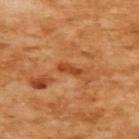The lesion was photographed on a routine skin check and not biopsied; there is no pathology result. Cropped from a whole-body photographic skin survey; the tile spans about 15 mm. A female patient approximately 55 years of age. The lesion is on the back. Automated image analysis of the tile measured a border-irregularity rating of about 3.5/10, internal color variation of about 0 on a 0–10 scale, and radial color variation of about 0. Measured at roughly 3 mm in maximum diameter.